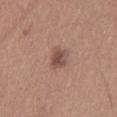  site: left thigh
  image:
    source: total-body photography crop
    field_of_view_mm: 15
  patient:
    sex: female
    age_approx: 45